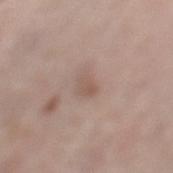The lesion was tiled from a total-body skin photograph and was not biopsied.
Automated image analysis of the tile measured an average lesion color of about L≈55 a*≈16 b*≈24 (CIELAB), roughly 7 lightness units darker than nearby skin, and a normalized border contrast of about 5. The analysis additionally found a nevus-likeness score of about 5/100 and a lesion-detection confidence of about 100/100.
The subject is a female in their mid-60s.
Located on the left lower leg.
The recorded lesion diameter is about 2.5 mm.
This is a white-light tile.
A region of skin cropped from a whole-body photographic capture, roughly 15 mm wide.